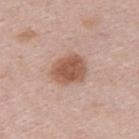Clinical summary: A male patient, approximately 25 years of age. This image is a 15 mm lesion crop taken from a total-body photograph. Located on the upper back. Measured at roughly 4 mm in maximum diameter. This is a white-light tile.